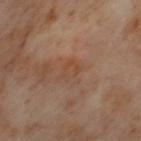Q: Was this lesion biopsied?
A: total-body-photography surveillance lesion; no biopsy
Q: What is the anatomic site?
A: the left thigh
Q: What is the lesion's diameter?
A: ≈2.5 mm
Q: Who is the patient?
A: female, aged 53 to 57
Q: What is the imaging modality?
A: total-body-photography crop, ~15 mm field of view